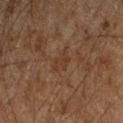- workup — total-body-photography surveillance lesion; no biopsy